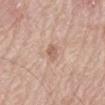Image and clinical context:
On the mid back. Approximately 2.5 mm at its widest. Imaged with white-light lighting. The lesion-visualizer software estimated a lesion color around L≈60 a*≈20 b*≈28 in CIELAB and a lesion-to-skin contrast of about 6.5 (normalized; higher = more distinct). It also reported a border-irregularity rating of about 2.5/10, a within-lesion color-variation index near 0.5/10, and peripheral color asymmetry of about 0. A male subject, aged around 80. A roughly 15 mm field-of-view crop from a total-body skin photograph.